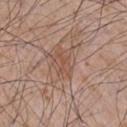No biopsy was performed on this lesion — it was imaged during a full skin examination and was not determined to be concerning. Located on the chest. A 15 mm close-up tile from a total-body photography series done for melanoma screening. The total-body-photography lesion software estimated an eccentricity of roughly 0.8 and two-axis asymmetry of about 0.35. And it measured an average lesion color of about L≈51 a*≈21 b*≈28 (CIELAB), about 6 CIELAB-L* units darker than the surrounding skin, and a normalized lesion–skin contrast near 5. This is a white-light tile. The recorded lesion diameter is about 3 mm. The subject is a male aged around 65.